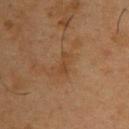workup = no biopsy performed (imaged during a skin exam) | illumination = cross-polarized | patient = male, about 55 years old | imaging modality = ~15 mm crop, total-body skin-cancer survey | anatomic site = the front of the torso | lesion diameter = about 2.5 mm.